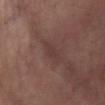{"biopsy_status": "not biopsied; imaged during a skin examination", "site": "leg", "lighting": "cross-polarized", "automated_metrics": {"area_mm2_approx": 3.5, "eccentricity": 0.9, "shape_asymmetry": 0.3, "border_irregularity_0_10": 3.0, "color_variation_0_10": 0.5, "peripheral_color_asymmetry": 0.0}, "image": {"source": "total-body photography crop", "field_of_view_mm": 15}}